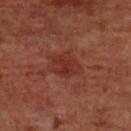Imaged during a routine full-body skin examination; the lesion was not biopsied and no histopathology is available.
The tile uses cross-polarized illumination.
On the front of the torso.
A male patient aged 58 to 62.
A region of skin cropped from a whole-body photographic capture, roughly 15 mm wide.